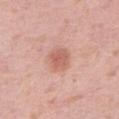This lesion was catalogued during total-body skin photography and was not selected for biopsy.
The recorded lesion diameter is about 3 mm.
A close-up tile cropped from a whole-body skin photograph, about 15 mm across.
From the right thigh.
The lesion-visualizer software estimated an area of roughly 6.5 mm² and a symmetry-axis asymmetry near 0.15. And it measured a color-variation rating of about 2.5/10 and a peripheral color-asymmetry measure near 1. The software also gave an automated nevus-likeness rating near 80 out of 100 and a lesion-detection confidence of about 100/100.
A female subject approximately 40 years of age.
Captured under white-light illumination.